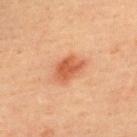| field | value |
|---|---|
| workup | no biopsy performed (imaged during a skin exam) |
| location | the upper back |
| image-analysis metrics | an outline eccentricity of about 0.75 (0 = round, 1 = elongated); a lesion color around L≈52 a*≈27 b*≈35 in CIELAB and about 11 CIELAB-L* units darker than the surrounding skin; a border-irregularity index near 2/10 and peripheral color asymmetry of about 0.5 |
| imaging modality | ~15 mm crop, total-body skin-cancer survey |
| subject | male, roughly 40 years of age |